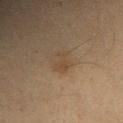The lesion was photographed on a routine skin check and not biopsied; there is no pathology result.
A 15 mm close-up tile from a total-body photography series done for melanoma screening.
From the right forearm.
A female subject aged approximately 40.